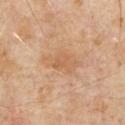Part of a total-body skin-imaging series; this lesion was reviewed on a skin check and was not flagged for biopsy.